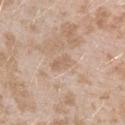Q: Is there a histopathology result?
A: catalogued during a skin exam; not biopsied
Q: How was this image acquired?
A: ~15 mm crop, total-body skin-cancer survey
Q: Lesion size?
A: ~3 mm (longest diameter)
Q: What is the anatomic site?
A: the left upper arm
Q: Who is the patient?
A: female, aged around 25
Q: What lighting was used for the tile?
A: white-light illumination